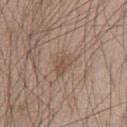Assessment:
No biopsy was performed on this lesion — it was imaged during a full skin examination and was not determined to be concerning.
Acquisition and patient details:
A male subject, roughly 45 years of age. The tile uses white-light illumination. On the chest. Cropped from a whole-body photographic skin survey; the tile spans about 15 mm. About 3 mm across. Automated tile analysis of the lesion measured a lesion area of about 6 mm², a shape eccentricity near 0.65, and a symmetry-axis asymmetry near 0.35. It also reported a lesion color around L≈52 a*≈16 b*≈27 in CIELAB, roughly 7 lightness units darker than nearby skin, and a normalized border contrast of about 5.5. The software also gave a lesion-detection confidence of about 100/100.